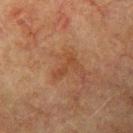Recorded during total-body skin imaging; not selected for excision or biopsy.
From the left upper arm.
The patient is a male aged 73 to 77.
Cropped from a whole-body photographic skin survey; the tile spans about 15 mm.